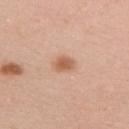Q: Was a biopsy performed?
A: no biopsy performed (imaged during a skin exam)
Q: What is the imaging modality?
A: total-body-photography crop, ~15 mm field of view
Q: Where on the body is the lesion?
A: the arm
Q: Patient demographics?
A: female, aged around 35